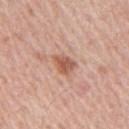image-analysis metrics = border irregularity of about 2.5 on a 0–10 scale, a within-lesion color-variation index near 4/10, and a peripheral color-asymmetry measure near 1.5; an automated nevus-likeness rating near 70 out of 100 and lesion-presence confidence of about 100/100 | size = ≈3 mm | subject = male, aged around 65 | site = the right upper arm | image source = total-body-photography crop, ~15 mm field of view.